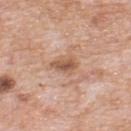Clinical impression: Captured during whole-body skin photography for melanoma surveillance; the lesion was not biopsied. Image and clinical context: A roughly 15 mm field-of-view crop from a total-body skin photograph. Located on the upper back. A male patient, in their 80s. The lesion-visualizer software estimated a lesion area of about 5 mm², an outline eccentricity of about 0.75 (0 = round, 1 = elongated), and a symmetry-axis asymmetry near 0.3. The software also gave a nevus-likeness score of about 0/100 and a detector confidence of about 100 out of 100 that the crop contains a lesion. Imaged with white-light lighting.